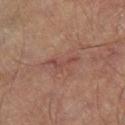The lesion was tiled from a total-body skin photograph and was not biopsied.
The lesion is on the leg.
Approximately 4 mm at its widest.
The subject is a male approximately 70 years of age.
Captured under cross-polarized illumination.
The total-body-photography lesion software estimated a lesion area of about 4 mm², an eccentricity of roughly 0.9, and a shape-asymmetry score of about 0.65 (0 = symmetric). It also reported a lesion color around L≈44 a*≈22 b*≈23 in CIELAB, about 7 CIELAB-L* units darker than the surrounding skin, and a normalized border contrast of about 6.
This image is a 15 mm lesion crop taken from a total-body photograph.